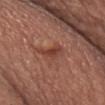Imaged during a routine full-body skin examination; the lesion was not biopsied and no histopathology is available.
A 15 mm close-up extracted from a 3D total-body photography capture.
This is a white-light tile.
From the front of the torso.
The recorded lesion diameter is about 3 mm.
A male patient, aged approximately 65.
The lesion-visualizer software estimated a lesion area of about 4.5 mm², an outline eccentricity of about 0.8 (0 = round, 1 = elongated), and two-axis asymmetry of about 0.45. The software also gave peripheral color asymmetry of about 1.